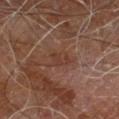Part of a total-body skin-imaging series; this lesion was reviewed on a skin check and was not flagged for biopsy.
Located on the leg.
A male subject aged approximately 60.
A lesion tile, about 15 mm wide, cut from a 3D total-body photograph.